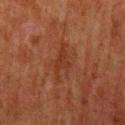Q: Is there a histopathology result?
A: total-body-photography surveillance lesion; no biopsy
Q: What is the anatomic site?
A: the mid back
Q: What is the imaging modality?
A: total-body-photography crop, ~15 mm field of view
Q: Who is the patient?
A: male, aged approximately 80